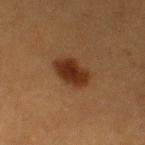No biopsy was performed on this lesion — it was imaged during a full skin examination and was not determined to be concerning. The subject is a female roughly 40 years of age. Automated tile analysis of the lesion measured a mean CIELAB color near L≈25 a*≈19 b*≈27, a lesion–skin lightness drop of about 10, and a normalized lesion–skin contrast near 11. The software also gave border irregularity of about 2 on a 0–10 scale, a color-variation rating of about 2.5/10, and radial color variation of about 0.5. And it measured an automated nevus-likeness rating near 100 out of 100 and a lesion-detection confidence of about 100/100. The lesion is on the left thigh. The tile uses cross-polarized illumination. About 4 mm across. A close-up tile cropped from a whole-body skin photograph, about 15 mm across.